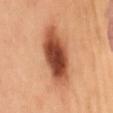Assessment:
Recorded during total-body skin imaging; not selected for excision or biopsy.
Context:
The lesion is located on the mid back. A female patient approximately 50 years of age. This image is a 15 mm lesion crop taken from a total-body photograph.